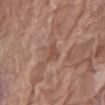Assessment: The lesion was tiled from a total-body skin photograph and was not biopsied. Clinical summary: About 2.5 mm across. On the leg. This is a white-light tile. A female patient approximately 75 years of age. A roughly 15 mm field-of-view crop from a total-body skin photograph.